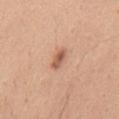Notes:
- notes — total-body-photography surveillance lesion; no biopsy
- image source — ~15 mm tile from a whole-body skin photo
- lesion size — about 3 mm
- location — the abdomen
- illumination — white-light
- subject — male, aged approximately 40